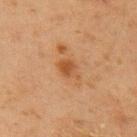Notes:
• follow-up — imaged on a skin check; not biopsied
• subject — male, aged 68–72
• lesion size — ≈2.5 mm
• body site — the right upper arm
• image — ~15 mm crop, total-body skin-cancer survey
• automated lesion analysis — an outline eccentricity of about 0.65 (0 = round, 1 = elongated) and a symmetry-axis asymmetry near 0.35; an average lesion color of about L≈43 a*≈21 b*≈34 (CIELAB), roughly 8 lightness units darker than nearby skin, and a normalized border contrast of about 7; a nevus-likeness score of about 45/100